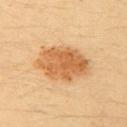Assessment:
The lesion was tiled from a total-body skin photograph and was not biopsied.
Clinical summary:
Imaged with cross-polarized lighting. The lesion is located on the left upper arm. The recorded lesion diameter is about 6 mm. A female subject, aged 33 to 37. A 15 mm close-up extracted from a 3D total-body photography capture. Automated image analysis of the tile measured a border-irregularity rating of about 1.5/10, internal color variation of about 4.5 on a 0–10 scale, and a peripheral color-asymmetry measure near 2. The analysis additionally found an automated nevus-likeness rating near 95 out of 100.